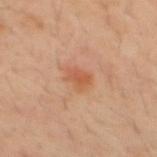biopsy_status: not biopsied; imaged during a skin examination
patient:
  sex: male
  age_approx: 30
image:
  source: total-body photography crop
  field_of_view_mm: 15
site: mid back
automated_metrics:
  area_mm2_approx: 4.0
  eccentricity: 0.65
  shape_asymmetry: 0.25
  cielab_L: 54
  cielab_a: 25
  cielab_b: 35
  vs_skin_darker_L: 8.0
  vs_skin_contrast_norm: 6.5
  color_variation_0_10: 4.0
  peripheral_color_asymmetry: 1.5